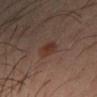No biopsy was performed on this lesion — it was imaged during a full skin examination and was not determined to be concerning. A 15 mm close-up tile from a total-body photography series done for melanoma screening. The recorded lesion diameter is about 3 mm. The patient is a male aged 38 to 42. The tile uses cross-polarized illumination. Located on the left forearm.